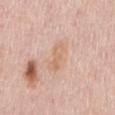Assessment:
Imaged during a routine full-body skin examination; the lesion was not biopsied and no histopathology is available.
Background:
Captured under white-light illumination. A 15 mm crop from a total-body photograph taken for skin-cancer surveillance. From the mid back. A female subject, in their 50s. An algorithmic analysis of the crop reported a lesion–skin lightness drop of about 6 and a lesion-to-skin contrast of about 5.5 (normalized; higher = more distinct). The software also gave a border-irregularity rating of about 2.5/10, internal color variation of about 2.5 on a 0–10 scale, and radial color variation of about 1. It also reported a classifier nevus-likeness of about 0/100 and a lesion-detection confidence of about 100/100.